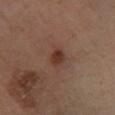Case summary:
- notes — no biopsy performed (imaged during a skin exam)
- acquisition — 15 mm crop, total-body photography
- anatomic site — the left lower leg
- lighting — cross-polarized
- TBP lesion metrics — a lesion area of about 4 mm², a shape eccentricity near 0.7, and a symmetry-axis asymmetry near 0.2; roughly 9 lightness units darker than nearby skin
- patient — male, about 55 years old